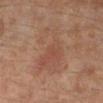Impression:
This lesion was catalogued during total-body skin photography and was not selected for biopsy.
Context:
Longest diameter approximately 6.5 mm. A close-up tile cropped from a whole-body skin photograph, about 15 mm across. The lesion is on the left leg. A male patient, aged 28–32. The total-body-photography lesion software estimated a lesion color around L≈48 a*≈20 b*≈28 in CIELAB and a lesion–skin lightness drop of about 5.